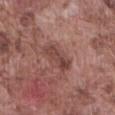- biopsy status · imaged on a skin check; not biopsied
- lighting · white-light illumination
- image source · 15 mm crop, total-body photography
- anatomic site · the abdomen
- patient · male, aged 73 to 77